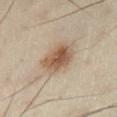Recorded during total-body skin imaging; not selected for excision or biopsy. A male subject roughly 50 years of age. A close-up tile cropped from a whole-body skin photograph, about 15 mm across. This is a cross-polarized tile. Located on the left lower leg. About 5 mm across.